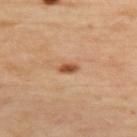The lesion is on the upper back. Captured under cross-polarized illumination. Measured at roughly 2.5 mm in maximum diameter. A female patient, in their mid-40s. A region of skin cropped from a whole-body photographic capture, roughly 15 mm wide.